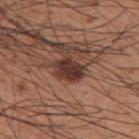The recorded lesion diameter is about 3 mm. A 15 mm close-up extracted from a 3D total-body photography capture. From the upper back. The patient is a male approximately 60 years of age. This is a white-light tile.